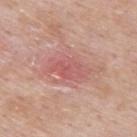Case summary:
• workup: total-body-photography surveillance lesion; no biopsy
• size: ≈5 mm
• patient: male, approximately 55 years of age
• location: the upper back
• image: ~15 mm tile from a whole-body skin photo
• tile lighting: white-light illumination